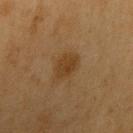notes: catalogued during a skin exam; not biopsied.